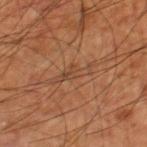A lesion tile, about 15 mm wide, cut from a 3D total-body photograph. Automated image analysis of the tile measured a mean CIELAB color near L≈44 a*≈21 b*≈32, roughly 6 lightness units darker than nearby skin, and a normalized border contrast of about 5. Located on the right thigh. A male patient aged approximately 60. This is a cross-polarized tile.